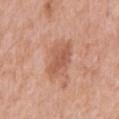biopsy status = total-body-photography surveillance lesion; no biopsy | lighting = white-light | acquisition = total-body-photography crop, ~15 mm field of view | automated lesion analysis = a border-irregularity rating of about 2.5/10, a color-variation rating of about 3/10, and a peripheral color-asymmetry measure near 1; a nevus-likeness score of about 15/100 and a detector confidence of about 100 out of 100 that the crop contains a lesion | body site = the abdomen | patient = male, in their 60s.